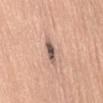Clinical impression:
The lesion was tiled from a total-body skin photograph and was not biopsied.
Clinical summary:
On the abdomen. This is a white-light tile. A roughly 15 mm field-of-view crop from a total-body skin photograph. About 3.5 mm across. The subject is a male aged 58–62. Automated tile analysis of the lesion measured an average lesion color of about L≈56 a*≈17 b*≈24 (CIELAB), roughly 15 lightness units darker than nearby skin, and a lesion-to-skin contrast of about 10.5 (normalized; higher = more distinct). The analysis additionally found a border-irregularity rating of about 3/10, a color-variation rating of about 2.5/10, and peripheral color asymmetry of about 0.5. The analysis additionally found a classifier nevus-likeness of about 30/100 and a lesion-detection confidence of about 100/100.